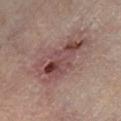Clinical impression: Recorded during total-body skin imaging; not selected for excision or biopsy. Image and clinical context: Approximately 7.5 mm at its widest. From the left lower leg. This is a cross-polarized tile. A male patient, aged 63 to 67. A 15 mm close-up extracted from a 3D total-body photography capture.